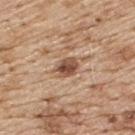workup: no biopsy performed (imaged during a skin exam)
automated metrics: a shape eccentricity near 0.8; a normalized lesion–skin contrast near 9; a border-irregularity rating of about 2/10 and a color-variation rating of about 5.5/10; an automated nevus-likeness rating near 50 out of 100 and lesion-presence confidence of about 100/100
size: about 4 mm
imaging modality: ~15 mm tile from a whole-body skin photo
site: the upper back
illumination: white-light
subject: male, aged approximately 70